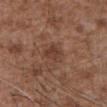  site: front of the torso
  patient:
    sex: male
    age_approx: 75
  image:
    source: total-body photography crop
    field_of_view_mm: 15
  lighting: white-light